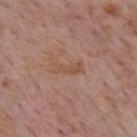Recorded during total-body skin imaging; not selected for excision or biopsy. Automated image analysis of the tile measured a border-irregularity rating of about 4.5/10, a color-variation rating of about 1/10, and radial color variation of about 0. Measured at roughly 3.5 mm in maximum diameter. A male subject aged around 75. Captured under white-light illumination. A lesion tile, about 15 mm wide, cut from a 3D total-body photograph. Located on the back.